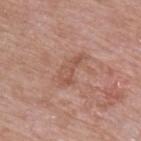Findings:
– follow-up · imaged on a skin check; not biopsied
– image · ~15 mm tile from a whole-body skin photo
– illumination · white-light
– anatomic site · the left upper arm
– subject · male, in their 60s